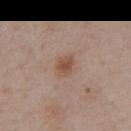workup = total-body-photography surveillance lesion; no biopsy
illumination = white-light illumination
body site = the front of the torso
size = ~2.5 mm (longest diameter)
subject = male, roughly 60 years of age
image = total-body-photography crop, ~15 mm field of view